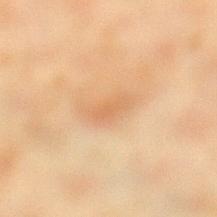workup: catalogued during a skin exam; not biopsied | subject: female, aged 38–42 | lighting: cross-polarized illumination | body site: the leg | acquisition: ~15 mm tile from a whole-body skin photo | diameter: ~4 mm (longest diameter) | automated lesion analysis: a footprint of about 6.5 mm², an outline eccentricity of about 0.9 (0 = round, 1 = elongated), and a shape-asymmetry score of about 0.3 (0 = symmetric); a lesion–skin lightness drop of about 7 and a normalized border contrast of about 5; a nevus-likeness score of about 0/100 and a detector confidence of about 100 out of 100 that the crop contains a lesion.